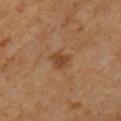The lesion was photographed on a routine skin check and not biopsied; there is no pathology result. This image is a 15 mm lesion crop taken from a total-body photograph. On the chest. The patient is a male roughly 60 years of age.